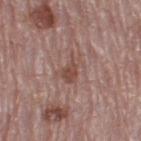{
  "biopsy_status": "not biopsied; imaged during a skin examination",
  "site": "right thigh",
  "patient": {
    "sex": "female",
    "age_approx": 65
  },
  "image": {
    "source": "total-body photography crop",
    "field_of_view_mm": 15
  },
  "lighting": "white-light",
  "lesion_size": {
    "long_diameter_mm_approx": 3.5
  }
}